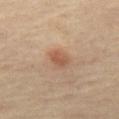Assessment: Captured during whole-body skin photography for melanoma surveillance; the lesion was not biopsied. Acquisition and patient details: Imaged with cross-polarized lighting. From the left thigh. A female patient aged 63–67. A 15 mm close-up tile from a total-body photography series done for melanoma screening. The lesion's longest dimension is about 2.5 mm.